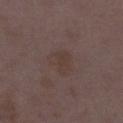A female subject in their mid-30s. A 15 mm close-up tile from a total-body photography series done for melanoma screening. Located on the left thigh. The total-body-photography lesion software estimated a border-irregularity index near 3/10 and peripheral color asymmetry of about 0.5. Measured at roughly 3.5 mm in maximum diameter.